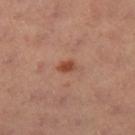Notes:
– biopsy status — catalogued during a skin exam; not biopsied
– diameter — ~2 mm (longest diameter)
– image source — total-body-photography crop, ~15 mm field of view
– subject — female, in their 40s
– image-analysis metrics — border irregularity of about 2.5 on a 0–10 scale and a color-variation rating of about 2/10; a nevus-likeness score of about 90/100 and lesion-presence confidence of about 100/100
– anatomic site — the right thigh
– illumination — cross-polarized